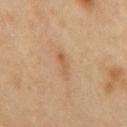Clinical impression: Recorded during total-body skin imaging; not selected for excision or biopsy. Background: Automated image analysis of the tile measured a lesion area of about 3 mm², an eccentricity of roughly 0.9, and a shape-asymmetry score of about 0.4 (0 = symmetric). It also reported border irregularity of about 4.5 on a 0–10 scale, a color-variation rating of about 0/10, and radial color variation of about 0. And it measured an automated nevus-likeness rating near 10 out of 100 and a lesion-detection confidence of about 100/100. From the mid back. A male subject about 55 years old. A roughly 15 mm field-of-view crop from a total-body skin photograph. Longest diameter approximately 3 mm. The tile uses cross-polarized illumination.